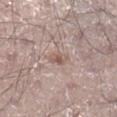The lesion was tiled from a total-body skin photograph and was not biopsied. About 2.5 mm across. A 15 mm close-up tile from a total-body photography series done for melanoma screening. This is a white-light tile. A male patient, about 30 years old. Located on the right lower leg.